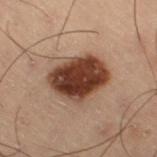The lesion was photographed on a routine skin check and not biopsied; there is no pathology result. The lesion's longest dimension is about 6 mm. Imaged with cross-polarized lighting. Located on the leg. Automated image analysis of the tile measured a lesion area of about 21 mm², a shape eccentricity near 0.65, and two-axis asymmetry of about 0.15. And it measured an average lesion color of about L≈31 a*≈18 b*≈23 (CIELAB), about 17 CIELAB-L* units darker than the surrounding skin, and a lesion-to-skin contrast of about 14.5 (normalized; higher = more distinct). The analysis additionally found a border-irregularity index near 2/10 and peripheral color asymmetry of about 1.5. A 15 mm close-up tile from a total-body photography series done for melanoma screening. The patient is a male aged 53 to 57.